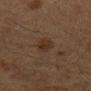Part of a total-body skin-imaging series; this lesion was reviewed on a skin check and was not flagged for biopsy.
A male subject aged approximately 65.
Longest diameter approximately 2.5 mm.
The total-body-photography lesion software estimated a lesion area of about 4 mm², a shape eccentricity near 0.8, and a symmetry-axis asymmetry near 0.15. The software also gave a border-irregularity rating of about 1.5/10, internal color variation of about 1.5 on a 0–10 scale, and radial color variation of about 0.5. The analysis additionally found a classifier nevus-likeness of about 55/100 and a lesion-detection confidence of about 100/100.
On the mid back.
Cropped from a whole-body photographic skin survey; the tile spans about 15 mm.
Captured under cross-polarized illumination.